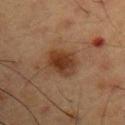Findings:
- patient — male, aged around 55
- body site — the arm
- image source — total-body-photography crop, ~15 mm field of view
- lesion size — ~3.5 mm (longest diameter)